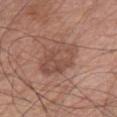No biopsy was performed on this lesion — it was imaged during a full skin examination and was not determined to be concerning. The tile uses white-light illumination. A close-up tile cropped from a whole-body skin photograph, about 15 mm across. Located on the chest. A male patient, aged around 70.